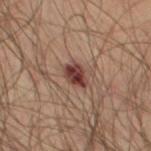<record>
  <biopsy_status>not biopsied; imaged during a skin examination</biopsy_status>
  <site>chest</site>
  <patient>
    <sex>male</sex>
    <age_approx>65</age_approx>
  </patient>
  <lesion_size>
    <long_diameter_mm_approx>3.0</long_diameter_mm_approx>
  </lesion_size>
  <automated_metrics>
    <area_mm2_approx>5.0</area_mm2_approx>
    <cielab_L>38</cielab_L>
    <cielab_a>22</cielab_a>
    <cielab_b>22</cielab_b>
    <vs_skin_darker_L>14.0</vs_skin_darker_L>
    <border_irregularity_0_10>2.5</border_irregularity_0_10>
    <color_variation_0_10>6.0</color_variation_0_10>
    <peripheral_color_asymmetry>2.0</peripheral_color_asymmetry>
    <nevus_likeness_0_100>20</nevus_likeness_0_100>
  </automated_metrics>
  <image>
    <source>total-body photography crop</source>
    <field_of_view_mm>15</field_of_view_mm>
  </image>
  <lighting>cross-polarized</lighting>
</record>